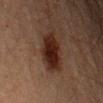- workup · total-body-photography surveillance lesion; no biopsy
- image-analysis metrics · a footprint of about 13 mm² and a symmetry-axis asymmetry near 0.15
- lesion diameter · ~6 mm (longest diameter)
- location · the left upper arm
- tile lighting · cross-polarized
- subject · male, approximately 60 years of age
- image · ~15 mm tile from a whole-body skin photo